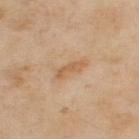The lesion was tiled from a total-body skin photograph and was not biopsied. A male patient approximately 55 years of age. The lesion's longest dimension is about 4 mm. Located on the upper back. A 15 mm close-up tile from a total-body photography series done for melanoma screening. The lesion-visualizer software estimated a lesion area of about 5 mm², an eccentricity of roughly 0.9, and a shape-asymmetry score of about 0.3 (0 = symmetric). The analysis additionally found about 8 CIELAB-L* units darker than the surrounding skin and a normalized lesion–skin contrast near 6. The analysis additionally found a classifier nevus-likeness of about 0/100 and lesion-presence confidence of about 100/100. This is a cross-polarized tile.